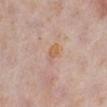Case summary:
* notes · catalogued during a skin exam; not biopsied
* size · ~2.5 mm (longest diameter)
* imaging modality · ~15 mm tile from a whole-body skin photo
* tile lighting · white-light illumination
* patient · female, aged approximately 60
* body site · the left lower leg
* automated lesion analysis · a lesion–skin lightness drop of about 6 and a lesion-to-skin contrast of about 7 (normalized; higher = more distinct); border irregularity of about 3 on a 0–10 scale, internal color variation of about 3.5 on a 0–10 scale, and a peripheral color-asymmetry measure near 1.5